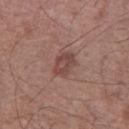A 15 mm crop from a total-body photograph taken for skin-cancer surveillance. Located on the left lower leg. Imaged with white-light lighting. About 3 mm across. The patient is a male aged 58–62.